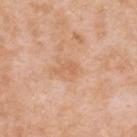Clinical impression:
The lesion was photographed on a routine skin check and not biopsied; there is no pathology result.
Clinical summary:
The lesion is on the upper back. Cropped from a whole-body photographic skin survey; the tile spans about 15 mm. The patient is a female aged 38–42. Imaged with white-light lighting. About 2.5 mm across.